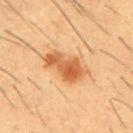subject: male, approximately 55 years of age | size: about 5.5 mm | lighting: cross-polarized | automated metrics: a footprint of about 11 mm², a shape eccentricity near 0.9, and a shape-asymmetry score of about 0.3 (0 = symmetric); a border-irregularity rating of about 4/10, internal color variation of about 4.5 on a 0–10 scale, and peripheral color asymmetry of about 1.5 | body site: the chest | imaging modality: ~15 mm tile from a whole-body skin photo.